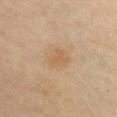Context: About 3 mm across. Captured under cross-polarized illumination. On the chest. A female subject, in their 40s. A 15 mm crop from a total-body photograph taken for skin-cancer surveillance.